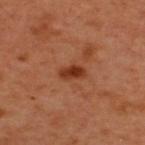A male patient, aged 48 to 52. Measured at roughly 3 mm in maximum diameter. The lesion-visualizer software estimated a footprint of about 4 mm², an eccentricity of roughly 0.85, and two-axis asymmetry of about 0.3. It also reported a mean CIELAB color near L≈37 a*≈29 b*≈34, about 11 CIELAB-L* units darker than the surrounding skin, and a lesion-to-skin contrast of about 9.5 (normalized; higher = more distinct). And it measured a classifier nevus-likeness of about 95/100 and a detector confidence of about 100 out of 100 that the crop contains a lesion. Captured under cross-polarized illumination. The lesion is located on the upper back. A close-up tile cropped from a whole-body skin photograph, about 15 mm across.